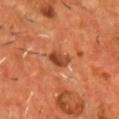site: the chest; patient: male, aged 53 to 57; acquisition: 15 mm crop, total-body photography.